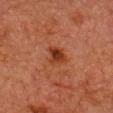Findings:
- workup · total-body-photography surveillance lesion; no biopsy
- illumination · cross-polarized
- patient · male, aged around 80
- anatomic site · the chest
- imaging modality · ~15 mm tile from a whole-body skin photo